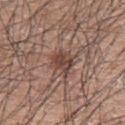Q: Was this lesion biopsied?
A: no biopsy performed (imaged during a skin exam)
Q: Lesion size?
A: ≈3 mm
Q: Illumination type?
A: white-light illumination
Q: Lesion location?
A: the left upper arm
Q: Patient demographics?
A: male, aged 68 to 72
Q: What did automated image analysis measure?
A: a lesion area of about 6.5 mm² and an eccentricity of roughly 0.4; an average lesion color of about L≈44 a*≈19 b*≈24 (CIELAB), about 10 CIELAB-L* units darker than the surrounding skin, and a normalized border contrast of about 8; a border-irregularity rating of about 2.5/10, internal color variation of about 3.5 on a 0–10 scale, and radial color variation of about 1; a nevus-likeness score of about 75/100 and a lesion-detection confidence of about 100/100
Q: What kind of image is this?
A: 15 mm crop, total-body photography